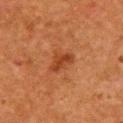notes = no biopsy performed (imaged during a skin exam)
size = ~3 mm (longest diameter)
image source = ~15 mm tile from a whole-body skin photo
automated lesion analysis = a lesion area of about 5 mm², an eccentricity of roughly 0.75, and two-axis asymmetry of about 0.4; a mean CIELAB color near L≈35 a*≈25 b*≈34, a lesion–skin lightness drop of about 8, and a normalized lesion–skin contrast near 7; a classifier nevus-likeness of about 15/100 and a lesion-detection confidence of about 100/100
illumination = cross-polarized illumination
subject = female, approximately 50 years of age
location = the chest